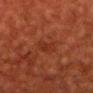The lesion was tiled from a total-body skin photograph and was not biopsied. Longest diameter approximately 2.5 mm. The lesion-visualizer software estimated a lesion area of about 4.5 mm² and a shape-asymmetry score of about 0.25 (0 = symmetric). The analysis additionally found a lesion color around L≈25 a*≈24 b*≈26 in CIELAB. From the head or neck. A 15 mm close-up tile from a total-body photography series done for melanoma screening. A male patient, aged around 60.